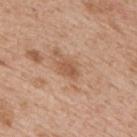workup = imaged on a skin check; not biopsied | location = the upper back | size = ≈3 mm | illumination = white-light | image source = ~15 mm tile from a whole-body skin photo | patient = male, approximately 65 years of age.